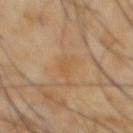<case>
  <biopsy_status>not biopsied; imaged during a skin examination</biopsy_status>
  <site>abdomen</site>
  <lighting>cross-polarized</lighting>
  <image>
    <source>total-body photography crop</source>
    <field_of_view_mm>15</field_of_view_mm>
  </image>
  <patient>
    <sex>male</sex>
    <age_approx>65</age_approx>
  </patient>
  <automated_metrics>
    <cielab_L>51</cielab_L>
    <cielab_a>17</cielab_a>
    <cielab_b>35</cielab_b>
    <border_irregularity_0_10>4.5</border_irregularity_0_10>
    <color_variation_0_10>0.0</color_variation_0_10>
    <peripheral_color_asymmetry>0.0</peripheral_color_asymmetry>
  </automated_metrics>
</case>